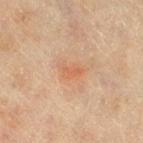Recorded during total-body skin imaging; not selected for excision or biopsy.
Automated tile analysis of the lesion measured a shape eccentricity near 0.8 and a shape-asymmetry score of about 0.45 (0 = symmetric). The software also gave an automated nevus-likeness rating near 0 out of 100 and a lesion-detection confidence of about 100/100.
Captured under cross-polarized illumination.
Measured at roughly 3 mm in maximum diameter.
From the right thigh.
A female subject approximately 55 years of age.
Cropped from a whole-body photographic skin survey; the tile spans about 15 mm.